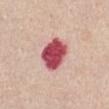notes: imaged on a skin check; not biopsied
subject: female, roughly 65 years of age
image source: 15 mm crop, total-body photography
location: the abdomen
automated metrics: a mean CIELAB color near L≈52 a*≈37 b*≈21, about 22 CIELAB-L* units darker than the surrounding skin, and a normalized border contrast of about 14; border irregularity of about 1.5 on a 0–10 scale and a color-variation rating of about 4/10
lighting: white-light